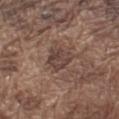Impression:
No biopsy was performed on this lesion — it was imaged during a full skin examination and was not determined to be concerning.